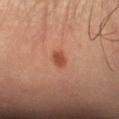The lesion was tiled from a total-body skin photograph and was not biopsied.
From the arm.
A roughly 15 mm field-of-view crop from a total-body skin photograph.
A male patient, approximately 50 years of age.
Captured under cross-polarized illumination.
The lesion-visualizer software estimated a within-lesion color-variation index near 1.5/10 and a peripheral color-asymmetry measure near 0.5. The analysis additionally found a nevus-likeness score of about 95/100 and lesion-presence confidence of about 100/100.